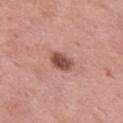Impression: This lesion was catalogued during total-body skin photography and was not selected for biopsy. Background: The subject is a female aged 48–52. An algorithmic analysis of the crop reported a border-irregularity index near 2/10. The software also gave an automated nevus-likeness rating near 95 out of 100. On the left thigh. Approximately 3 mm at its widest. A roughly 15 mm field-of-view crop from a total-body skin photograph.